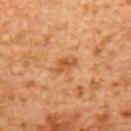Findings:
* follow-up — total-body-photography surveillance lesion; no biopsy
* imaging modality — ~15 mm tile from a whole-body skin photo
* lighting — cross-polarized
* site — the back
* subject — female, roughly 55 years of age
* lesion diameter — about 3 mm